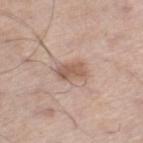The lesion was photographed on a routine skin check and not biopsied; there is no pathology result.
The lesion is located on the right lower leg.
This image is a 15 mm lesion crop taken from a total-body photograph.
Longest diameter approximately 3.5 mm.
The total-body-photography lesion software estimated an eccentricity of roughly 0.85 and a shape-asymmetry score of about 0.25 (0 = symmetric). It also reported a mean CIELAB color near L≈56 a*≈19 b*≈28, a lesion–skin lightness drop of about 11, and a normalized border contrast of about 7.5. And it measured a border-irregularity index near 3/10, a color-variation rating of about 2.5/10, and a peripheral color-asymmetry measure near 1. It also reported a nevus-likeness score of about 75/100 and a detector confidence of about 100 out of 100 that the crop contains a lesion.
The patient is a male approximately 60 years of age.